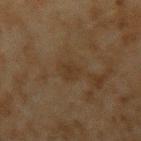Assessment: This lesion was catalogued during total-body skin photography and was not selected for biopsy. Image and clinical context: An algorithmic analysis of the crop reported a lesion area of about 4 mm², a shape eccentricity near 0.8, and a symmetry-axis asymmetry near 0.25. And it measured an average lesion color of about L≈27 a*≈12 b*≈24 (CIELAB) and a lesion–skin lightness drop of about 4. The analysis additionally found a border-irregularity rating of about 3/10, internal color variation of about 1 on a 0–10 scale, and a peripheral color-asymmetry measure near 0.5. The analysis additionally found an automated nevus-likeness rating near 0 out of 100 and a lesion-detection confidence of about 100/100. A 15 mm crop from a total-body photograph taken for skin-cancer surveillance. The lesion is on the left upper arm. Measured at roughly 3 mm in maximum diameter. A male subject aged approximately 45. Imaged with cross-polarized lighting.